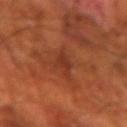Impression: Part of a total-body skin-imaging series; this lesion was reviewed on a skin check and was not flagged for biopsy. Image and clinical context: A male patient, in their 70s. On the left forearm. A 15 mm close-up tile from a total-body photography series done for melanoma screening.